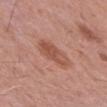Q: Was this lesion biopsied?
A: total-body-photography surveillance lesion; no biopsy
Q: What is the lesion's diameter?
A: about 4.5 mm
Q: How was this image acquired?
A: 15 mm crop, total-body photography
Q: Illumination type?
A: white-light illumination
Q: Patient demographics?
A: female, in their mid-60s
Q: Where on the body is the lesion?
A: the arm